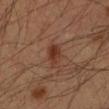<case>
<biopsy_status>not biopsied; imaged during a skin examination</biopsy_status>
<automated_metrics>
  <area_mm2_approx>4.0</area_mm2_approx>
  <eccentricity>0.7</eccentricity>
  <shape_asymmetry>0.2</shape_asymmetry>
  <lesion_detection_confidence_0_100>100</lesion_detection_confidence_0_100>
</automated_metrics>
<site>left forearm</site>
<lighting>cross-polarized</lighting>
<image>
  <source>total-body photography crop</source>
  <field_of_view_mm>15</field_of_view_mm>
</image>
<lesion_size>
  <long_diameter_mm_approx>2.5</long_diameter_mm_approx>
</lesion_size>
<patient>
  <sex>male</sex>
  <age_approx>35</age_approx>
</patient>
</case>